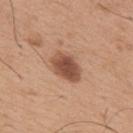Assessment:
This lesion was catalogued during total-body skin photography and was not selected for biopsy.
Background:
This image is a 15 mm lesion crop taken from a total-body photograph. Imaged with white-light lighting. A male patient aged around 65. The lesion is located on the back.